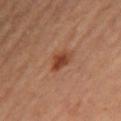Part of a total-body skin-imaging series; this lesion was reviewed on a skin check and was not flagged for biopsy.
Located on the right thigh.
Longest diameter approximately 3 mm.
The total-body-photography lesion software estimated a footprint of about 4.5 mm², a shape eccentricity near 0.75, and a symmetry-axis asymmetry near 0.25. And it measured an average lesion color of about L≈43 a*≈26 b*≈33 (CIELAB) and a normalized border contrast of about 9. It also reported a color-variation rating of about 2.5/10 and a peripheral color-asymmetry measure near 0.5. The software also gave a nevus-likeness score of about 90/100 and a lesion-detection confidence of about 100/100.
A female subject, aged around 35.
Cropped from a total-body skin-imaging series; the visible field is about 15 mm.
Imaged with cross-polarized lighting.